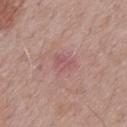This lesion was catalogued during total-body skin photography and was not selected for biopsy.